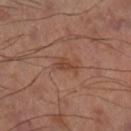Assessment: The lesion was photographed on a routine skin check and not biopsied; there is no pathology result. Acquisition and patient details: Cropped from a total-body skin-imaging series; the visible field is about 15 mm. From the right thigh.